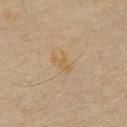Imaged during a routine full-body skin examination; the lesion was not biopsied and no histopathology is available.
A 15 mm crop from a total-body photograph taken for skin-cancer surveillance.
The lesion is on the chest.
A male subject in their mid- to late 60s.
Automated tile analysis of the lesion measured internal color variation of about 1 on a 0–10 scale and peripheral color asymmetry of about 0.5.
Captured under cross-polarized illumination.
Approximately 2.5 mm at its widest.